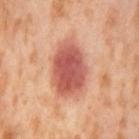Recorded during total-body skin imaging; not selected for excision or biopsy.
This image is a 15 mm lesion crop taken from a total-body photograph.
On the left thigh.
An algorithmic analysis of the crop reported a color-variation rating of about 4.5/10 and a peripheral color-asymmetry measure near 1.5.
Imaged with cross-polarized lighting.
Longest diameter approximately 6.5 mm.
A female patient, approximately 55 years of age.